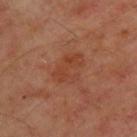No biopsy was performed on this lesion — it was imaged during a full skin examination and was not determined to be concerning.
A male subject, aged around 65.
Located on the upper back.
A region of skin cropped from a whole-body photographic capture, roughly 15 mm wide.
This is a cross-polarized tile.
An algorithmic analysis of the crop reported a footprint of about 9 mm², an eccentricity of roughly 0.75, and two-axis asymmetry of about 0.4. It also reported border irregularity of about 5.5 on a 0–10 scale, a within-lesion color-variation index near 3/10, and peripheral color asymmetry of about 1. The software also gave a nevus-likeness score of about 5/100 and lesion-presence confidence of about 100/100.